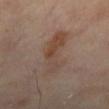Captured during whole-body skin photography for melanoma surveillance; the lesion was not biopsied.